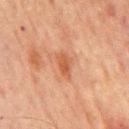Clinical impression: The lesion was photographed on a routine skin check and not biopsied; there is no pathology result. Acquisition and patient details: Located on the back. An algorithmic analysis of the crop reported an outline eccentricity of about 0.85 (0 = round, 1 = elongated) and a shape-asymmetry score of about 0.2 (0 = symmetric). It also reported a lesion color around L≈45 a*≈23 b*≈31 in CIELAB, roughly 8 lightness units darker than nearby skin, and a normalized lesion–skin contrast near 6.5. A 15 mm close-up tile from a total-body photography series done for melanoma screening. The patient is a male in their mid- to late 60s.